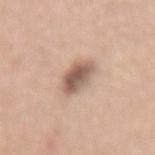The lesion was tiled from a total-body skin photograph and was not biopsied. Longest diameter approximately 4 mm. Located on the mid back. A lesion tile, about 15 mm wide, cut from a 3D total-body photograph. The tile uses white-light illumination. A female patient aged around 55.